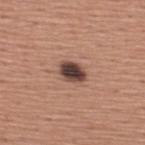Case summary:
• biopsy status — catalogued during a skin exam; not biopsied
• lesion size — ~3.5 mm (longest diameter)
• subject — male, aged approximately 45
• site — the upper back
• image source — 15 mm crop, total-body photography
• tile lighting — white-light